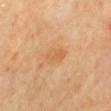| feature | finding |
|---|---|
| notes | catalogued during a skin exam; not biopsied |
| subject | male, about 70 years old |
| body site | the mid back |
| image source | 15 mm crop, total-body photography |